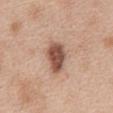<tbp_lesion>
  <biopsy_status>not biopsied; imaged during a skin examination</biopsy_status>
  <automated_metrics>
    <area_mm2_approx>9.5</area_mm2_approx>
    <eccentricity>0.8</eccentricity>
    <shape_asymmetry>0.2</shape_asymmetry>
  </automated_metrics>
  <image>
    <source>total-body photography crop</source>
    <field_of_view_mm>15</field_of_view_mm>
  </image>
  <patient>
    <sex>female</sex>
    <age_approx>40</age_approx>
  </patient>
  <site>abdomen</site>
  <lesion_size>
    <long_diameter_mm_approx>4.5</long_diameter_mm_approx>
  </lesion_size>
</tbp_lesion>